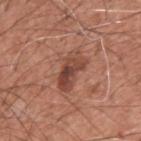Recorded during total-body skin imaging; not selected for excision or biopsy. A male subject aged 58–62. Measured at roughly 4.5 mm in maximum diameter. Located on the upper back. Cropped from a whole-body photographic skin survey; the tile spans about 15 mm.